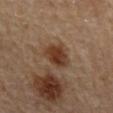This lesion was catalogued during total-body skin photography and was not selected for biopsy. The subject is a male aged 83 or older. The lesion is located on the mid back. About 4 mm across. This is a cross-polarized tile. A region of skin cropped from a whole-body photographic capture, roughly 15 mm wide.